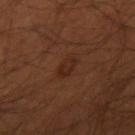The lesion was photographed on a routine skin check and not biopsied; there is no pathology result. The lesion's longest dimension is about 2.5 mm. A male patient, in their mid-30s. Cropped from a total-body skin-imaging series; the visible field is about 15 mm. The tile uses cross-polarized illumination. The lesion is located on the right upper arm.